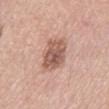Notes:
– follow-up: catalogued during a skin exam; not biopsied
– location: the left lower leg
– image: 15 mm crop, total-body photography
– subject: female, aged 63–67